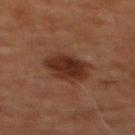Imaged during a routine full-body skin examination; the lesion was not biopsied and no histopathology is available.
A roughly 15 mm field-of-view crop from a total-body skin photograph.
The patient is a male in their 60s.
The lesion is on the front of the torso.